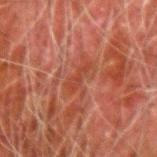follow-up — total-body-photography surveillance lesion; no biopsy | anatomic site — the right forearm | lesion size — ~3.5 mm (longest diameter) | imaging modality — total-body-photography crop, ~15 mm field of view | patient — male, aged 78–82 | automated metrics — a footprint of about 3.5 mm², a shape eccentricity near 0.95, and a shape-asymmetry score of about 0.4 (0 = symmetric); roughly 5 lightness units darker than nearby skin and a lesion-to-skin contrast of about 5.5 (normalized; higher = more distinct).